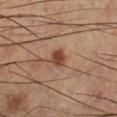The lesion was photographed on a routine skin check and not biopsied; there is no pathology result. This is a cross-polarized tile. A male subject, aged around 65. About 2.5 mm across. Located on the right lower leg. Cropped from a whole-body photographic skin survey; the tile spans about 15 mm. An algorithmic analysis of the crop reported a footprint of about 3.5 mm², a shape eccentricity near 0.55, and a shape-asymmetry score of about 0.25 (0 = symmetric). The analysis additionally found a border-irregularity rating of about 2/10, internal color variation of about 2 on a 0–10 scale, and peripheral color asymmetry of about 0.5. The analysis additionally found a classifier nevus-likeness of about 95/100 and a detector confidence of about 100 out of 100 that the crop contains a lesion.